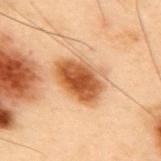| feature | finding |
|---|---|
| workup | no biopsy performed (imaged during a skin exam) |
| diameter | ≈5.5 mm |
| subject | male, aged 53–57 |
| automated metrics | a lesion area of about 17 mm² and an eccentricity of roughly 0.75; a border-irregularity index near 2.5/10, a color-variation rating of about 5.5/10, and radial color variation of about 1.5; a classifier nevus-likeness of about 100/100 and a lesion-detection confidence of about 100/100 |
| site | the mid back |
| tile lighting | cross-polarized |
| acquisition | ~15 mm crop, total-body skin-cancer survey |